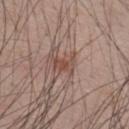* biopsy status: no biopsy performed (imaged during a skin exam)
* lighting: white-light illumination
* subject: male, aged approximately 35
* TBP lesion metrics: a footprint of about 3.5 mm², a shape eccentricity near 0.85, and a symmetry-axis asymmetry near 0.3; border irregularity of about 4 on a 0–10 scale, a within-lesion color-variation index near 1/10, and radial color variation of about 0; an automated nevus-likeness rating near 75 out of 100 and a detector confidence of about 100 out of 100 that the crop contains a lesion
* acquisition: total-body-photography crop, ~15 mm field of view
* lesion diameter: ~2.5 mm (longest diameter)
* body site: the back